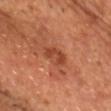Q: Was this lesion biopsied?
A: total-body-photography surveillance lesion; no biopsy
Q: What did automated image analysis measure?
A: roughly 9 lightness units darker than nearby skin and a normalized lesion–skin contrast near 7; an automated nevus-likeness rating near 15 out of 100 and lesion-presence confidence of about 100/100
Q: Lesion size?
A: about 3.5 mm
Q: How was this image acquired?
A: ~15 mm tile from a whole-body skin photo
Q: What are the patient's age and sex?
A: male, in their mid-60s
Q: What lighting was used for the tile?
A: cross-polarized